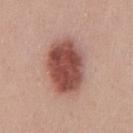Assessment: No biopsy was performed on this lesion — it was imaged during a full skin examination and was not determined to be concerning. Acquisition and patient details: A 15 mm close-up extracted from a 3D total-body photography capture. The subject is a male aged around 25. The tile uses white-light illumination. Approximately 7 mm at its widest. Located on the chest. The lesion-visualizer software estimated a nevus-likeness score of about 100/100 and a lesion-detection confidence of about 100/100.